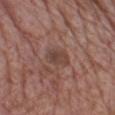No biopsy was performed on this lesion — it was imaged during a full skin examination and was not determined to be concerning.
A region of skin cropped from a whole-body photographic capture, roughly 15 mm wide.
A male subject in their mid- to late 60s.
On the chest.